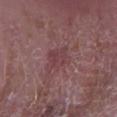Q: Is there a histopathology result?
A: no biopsy performed (imaged during a skin exam)
Q: Who is the patient?
A: male, in their mid-60s
Q: Where on the body is the lesion?
A: the right forearm
Q: Automated lesion metrics?
A: about 6 CIELAB-L* units darker than the surrounding skin; border irregularity of about 3.5 on a 0–10 scale, internal color variation of about 2.5 on a 0–10 scale, and peripheral color asymmetry of about 1; a nevus-likeness score of about 0/100
Q: What lighting was used for the tile?
A: white-light
Q: How was this image acquired?
A: 15 mm crop, total-body photography